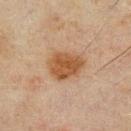patient:
  sex: male
  age_approx: 70
site: chest
lighting: cross-polarized
lesion_size:
  long_diameter_mm_approx: 4.5
image:
  source: total-body photography crop
  field_of_view_mm: 15